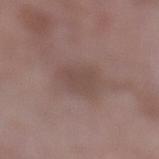Q: Was this lesion biopsied?
A: catalogued during a skin exam; not biopsied
Q: Lesion size?
A: about 4.5 mm
Q: What kind of image is this?
A: ~15 mm tile from a whole-body skin photo
Q: Automated lesion metrics?
A: lesion-presence confidence of about 100/100
Q: Where on the body is the lesion?
A: the left lower leg
Q: How was the tile lit?
A: white-light illumination
Q: What are the patient's age and sex?
A: female, aged approximately 70